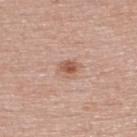This lesion was catalogued during total-body skin photography and was not selected for biopsy.
An algorithmic analysis of the crop reported an outline eccentricity of about 0.55 (0 = round, 1 = elongated).
The patient is a female approximately 50 years of age.
The tile uses white-light illumination.
A lesion tile, about 15 mm wide, cut from a 3D total-body photograph.
From the upper back.